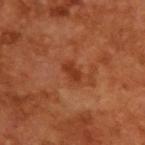No biopsy was performed on this lesion — it was imaged during a full skin examination and was not determined to be concerning. The lesion's longest dimension is about 2.5 mm. A region of skin cropped from a whole-body photographic capture, roughly 15 mm wide. The subject is a male aged 63–67. The tile uses cross-polarized illumination.